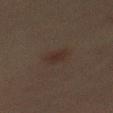The lesion was tiled from a total-body skin photograph and was not biopsied. A male patient roughly 60 years of age. The lesion is on the back. This image is a 15 mm lesion crop taken from a total-body photograph.